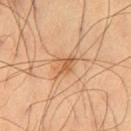| field | value |
|---|---|
| follow-up | total-body-photography surveillance lesion; no biopsy |
| acquisition | ~15 mm tile from a whole-body skin photo |
| subject | male, about 60 years old |
| location | the left thigh |